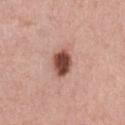body site: the mid back
lesion diameter: ≈3.5 mm
lighting: white-light
automated lesion analysis: two-axis asymmetry of about 0.2; about 19 CIELAB-L* units darker than the surrounding skin and a lesion-to-skin contrast of about 12.5 (normalized; higher = more distinct); border irregularity of about 1.5 on a 0–10 scale, a color-variation rating of about 5/10, and a peripheral color-asymmetry measure near 1.5; a nevus-likeness score of about 100/100
image: ~15 mm crop, total-body skin-cancer survey
patient: male, aged 38–42